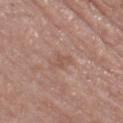Impression: No biopsy was performed on this lesion — it was imaged during a full skin examination and was not determined to be concerning. Acquisition and patient details: Measured at roughly 2.5 mm in maximum diameter. A region of skin cropped from a whole-body photographic capture, roughly 15 mm wide. A male patient, roughly 85 years of age or older. Automated tile analysis of the lesion measured a lesion color around L≈53 a*≈20 b*≈27 in CIELAB, roughly 6 lightness units darker than nearby skin, and a normalized border contrast of about 5. And it measured border irregularity of about 4 on a 0–10 scale. The analysis additionally found a classifier nevus-likeness of about 0/100 and a lesion-detection confidence of about 100/100. Located on the right thigh. Imaged with white-light lighting.